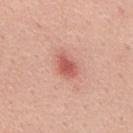workup = catalogued during a skin exam; not biopsied
anatomic site = the mid back
patient = male, in their mid-50s
lesion size = about 3 mm
automated lesion analysis = a lesion area of about 5 mm², an eccentricity of roughly 0.75, and a symmetry-axis asymmetry near 0.25
image = total-body-photography crop, ~15 mm field of view
illumination = white-light illumination